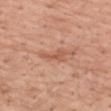- notes · no biopsy performed (imaged during a skin exam)
- site · the arm
- tile lighting · white-light illumination
- imaging modality · ~15 mm crop, total-body skin-cancer survey
- subject · female, in their mid-60s
- size · ~3.5 mm (longest diameter)